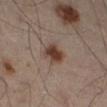<case>
  <biopsy_status>not biopsied; imaged during a skin examination</biopsy_status>
  <site>leg</site>
  <lesion_size>
    <long_diameter_mm_approx>3.0</long_diameter_mm_approx>
  </lesion_size>
  <patient>
    <sex>male</sex>
    <age_approx>50</age_approx>
  </patient>
  <image>
    <source>total-body photography crop</source>
    <field_of_view_mm>15</field_of_view_mm>
  </image>
</case>